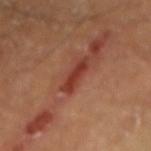Findings:
• biopsy status — imaged on a skin check; not biopsied
• tile lighting — cross-polarized
• subject — male, aged 58–62
• anatomic site — the right forearm
• imaging modality — ~15 mm tile from a whole-body skin photo
• size — ~3.5 mm (longest diameter)
• TBP lesion metrics — a border-irregularity index near 4/10